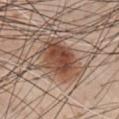Clinical impression:
Imaged during a routine full-body skin examination; the lesion was not biopsied and no histopathology is available.
Clinical summary:
This image is a 15 mm lesion crop taken from a total-body photograph. A male patient, aged 58 to 62. Automated tile analysis of the lesion measured border irregularity of about 3 on a 0–10 scale, a within-lesion color-variation index near 6/10, and radial color variation of about 2. On the chest.